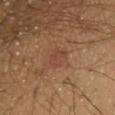Assessment: Part of a total-body skin-imaging series; this lesion was reviewed on a skin check and was not flagged for biopsy. Context: The total-body-photography lesion software estimated an area of roughly 4.5 mm², a shape eccentricity near 0.65, and two-axis asymmetry of about 0.35. The analysis additionally found an average lesion color of about L≈42 a*≈23 b*≈28 (CIELAB), about 5 CIELAB-L* units darker than the surrounding skin, and a lesion-to-skin contrast of about 5 (normalized; higher = more distinct). The software also gave a border-irregularity rating of about 3.5/10, a color-variation rating of about 1.5/10, and radial color variation of about 0.5. And it measured a lesion-detection confidence of about 100/100. The tile uses cross-polarized illumination. Measured at roughly 2.5 mm in maximum diameter. The lesion is located on the chest. A close-up tile cropped from a whole-body skin photograph, about 15 mm across. The subject is a male in their 40s.